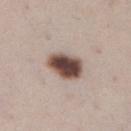Captured during whole-body skin photography for melanoma surveillance; the lesion was not biopsied.
Automated image analysis of the tile measured a footprint of about 12 mm², a shape eccentricity near 0.75, and a symmetry-axis asymmetry near 0.15.
Measured at roughly 4.5 mm in maximum diameter.
From the right lower leg.
A female subject aged 38 to 42.
The tile uses white-light illumination.
A roughly 15 mm field-of-view crop from a total-body skin photograph.